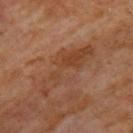<lesion>
  <lighting>cross-polarized</lighting>
  <site>back</site>
  <automated_metrics>
    <shape_asymmetry>0.6</shape_asymmetry>
    <color_variation_0_10>4.5</color_variation_0_10>
    <peripheral_color_asymmetry>1.5</peripheral_color_asymmetry>
  </automated_metrics>
  <image>
    <source>total-body photography crop</source>
    <field_of_view_mm>15</field_of_view_mm>
  </image>
  <patient>
    <sex>male</sex>
    <age_approx>60</age_approx>
  </patient>
  <lesion_size>
    <long_diameter_mm_approx>8.0</long_diameter_mm_approx>
  </lesion_size>
</lesion>